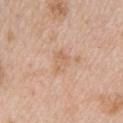biopsy status = no biopsy performed (imaged during a skin exam) | image source = ~15 mm tile from a whole-body skin photo | subject = female, about 45 years old | automated metrics = a border-irregularity index near 3/10, a color-variation rating of about 2.5/10, and a peripheral color-asymmetry measure near 1; an automated nevus-likeness rating near 0 out of 100 and a lesion-detection confidence of about 100/100 | lighting = white-light | diameter = ≈2.5 mm | body site = the upper back.